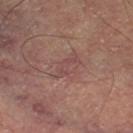notes: total-body-photography surveillance lesion; no biopsy | illumination: cross-polarized illumination | imaging modality: total-body-photography crop, ~15 mm field of view | site: the left thigh | patient: male, aged approximately 65 | image-analysis metrics: an automated nevus-likeness rating near 0 out of 100.